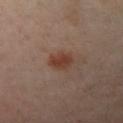Imaged during a routine full-body skin examination; the lesion was not biopsied and no histopathology is available. A female subject aged approximately 40. On the left arm. A roughly 15 mm field-of-view crop from a total-body skin photograph. The lesion-visualizer software estimated a lesion color around L≈39 a*≈21 b*≈27 in CIELAB, about 9 CIELAB-L* units darker than the surrounding skin, and a normalized border contrast of about 8.5. It also reported a nevus-likeness score of about 95/100 and lesion-presence confidence of about 100/100.